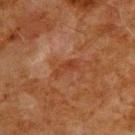Q: Was this lesion biopsied?
A: catalogued during a skin exam; not biopsied
Q: What are the patient's age and sex?
A: male, approximately 80 years of age
Q: How was this image acquired?
A: ~15 mm crop, total-body skin-cancer survey
Q: Lesion location?
A: the back